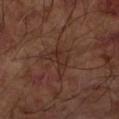Part of a total-body skin-imaging series; this lesion was reviewed on a skin check and was not flagged for biopsy. The subject is a male aged 63 to 67. The tile uses cross-polarized illumination. This image is a 15 mm lesion crop taken from a total-body photograph. The recorded lesion diameter is about 3.5 mm. The lesion is on the left forearm.